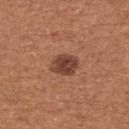<case>
<biopsy_status>not biopsied; imaged during a skin examination</biopsy_status>
<lighting>white-light</lighting>
<image>
  <source>total-body photography crop</source>
  <field_of_view_mm>15</field_of_view_mm>
</image>
<patient>
  <sex>female</sex>
  <age_approx>35</age_approx>
</patient>
<site>upper back</site>
</case>